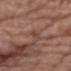{
  "biopsy_status": "not biopsied; imaged during a skin examination",
  "site": "right upper arm",
  "patient": {
    "sex": "male",
    "age_approx": 70
  },
  "image": {
    "source": "total-body photography crop",
    "field_of_view_mm": 15
  }
}